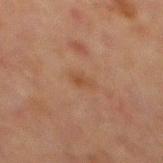Context: From the front of the torso. About 2.5 mm across. The subject is a male approximately 65 years of age. A 15 mm close-up tile from a total-body photography series done for melanoma screening. Automated image analysis of the tile measured an outline eccentricity of about 0.85 (0 = round, 1 = elongated) and two-axis asymmetry of about 0.35. It also reported a border-irregularity index near 3.5/10, a color-variation rating of about 1/10, and radial color variation of about 0. The analysis additionally found a nevus-likeness score of about 0/100 and a detector confidence of about 100 out of 100 that the crop contains a lesion.